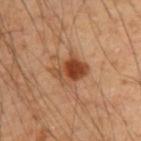Assessment:
No biopsy was performed on this lesion — it was imaged during a full skin examination and was not determined to be concerning.
Acquisition and patient details:
Longest diameter approximately 4 mm. The tile uses cross-polarized illumination. A 15 mm close-up extracted from a 3D total-body photography capture. The lesion-visualizer software estimated a mean CIELAB color near L≈44 a*≈24 b*≈34, about 13 CIELAB-L* units darker than the surrounding skin, and a normalized lesion–skin contrast near 10. And it measured lesion-presence confidence of about 100/100. Located on the upper back. A male patient, approximately 55 years of age.